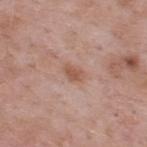<tbp_lesion>
<biopsy_status>not biopsied; imaged during a skin examination</biopsy_status>
<lighting>white-light</lighting>
<patient>
  <sex>male</sex>
  <age_approx>55</age_approx>
</patient>
<image>
  <source>total-body photography crop</source>
  <field_of_view_mm>15</field_of_view_mm>
</image>
<site>upper back</site>
</tbp_lesion>